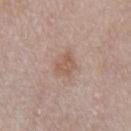Q: Was a biopsy performed?
A: imaged on a skin check; not biopsied
Q: What is the anatomic site?
A: the chest
Q: How was this image acquired?
A: ~15 mm crop, total-body skin-cancer survey
Q: Who is the patient?
A: male, in their mid- to late 50s
Q: What is the lesion's diameter?
A: ~3 mm (longest diameter)